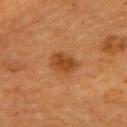This is a cross-polarized tile.
The recorded lesion diameter is about 3.5 mm.
The lesion is located on the upper back.
The patient is a female roughly 55 years of age.
Cropped from a total-body skin-imaging series; the visible field is about 15 mm.